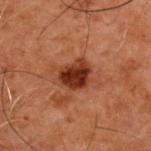No biopsy was performed on this lesion — it was imaged during a full skin examination and was not determined to be concerning. The patient is a male approximately 50 years of age. This image is a 15 mm lesion crop taken from a total-body photograph. From the upper back.